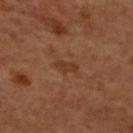* biopsy status: imaged on a skin check; not biopsied
* subject: male, roughly 70 years of age
* acquisition: total-body-photography crop, ~15 mm field of view
* location: the upper back